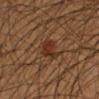follow-up: no biopsy performed (imaged during a skin exam)
location: the left forearm
subject: male, approximately 40 years of age
image-analysis metrics: a lesion area of about 5.5 mm², a shape eccentricity near 0.65, and a shape-asymmetry score of about 0.3 (0 = symmetric); about 8 CIELAB-L* units darker than the surrounding skin and a normalized lesion–skin contrast near 8; border irregularity of about 3.5 on a 0–10 scale and a within-lesion color-variation index near 2/10
size: ≈3 mm
lighting: cross-polarized illumination
imaging modality: 15 mm crop, total-body photography